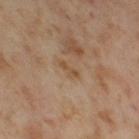The lesion was photographed on a routine skin check and not biopsied; there is no pathology result. A female patient, roughly 55 years of age. The recorded lesion diameter is about 2.5 mm. Captured under cross-polarized illumination. A 15 mm close-up tile from a total-body photography series done for melanoma screening. Located on the right thigh.